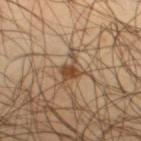Impression:
The lesion was photographed on a routine skin check and not biopsied; there is no pathology result.
Clinical summary:
Cropped from a total-body skin-imaging series; the visible field is about 15 mm. The lesion is located on the right thigh. The patient is a male aged approximately 45.